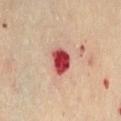Impression:
Captured during whole-body skin photography for melanoma surveillance; the lesion was not biopsied.
Clinical summary:
The lesion's longest dimension is about 3.5 mm. Captured under cross-polarized illumination. A female subject approximately 60 years of age. Cropped from a total-body skin-imaging series; the visible field is about 15 mm. The lesion is located on the abdomen.